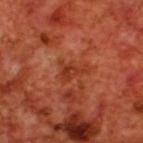Q: Was a biopsy performed?
A: catalogued during a skin exam; not biopsied
Q: Automated lesion metrics?
A: a mean CIELAB color near L≈37 a*≈31 b*≈35 and a normalized border contrast of about 6; border irregularity of about 6 on a 0–10 scale and internal color variation of about 2.5 on a 0–10 scale
Q: How was this image acquired?
A: 15 mm crop, total-body photography
Q: Patient demographics?
A: male, aged 68–72
Q: What lighting was used for the tile?
A: cross-polarized
Q: Lesion location?
A: the back